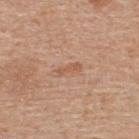Q: Was a biopsy performed?
A: imaged on a skin check; not biopsied
Q: Lesion size?
A: ~3 mm (longest diameter)
Q: What is the imaging modality?
A: ~15 mm crop, total-body skin-cancer survey
Q: What is the anatomic site?
A: the back
Q: Patient demographics?
A: male, aged around 55